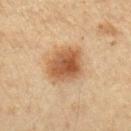– workup — imaged on a skin check; not biopsied
– automated lesion analysis — a lesion color around L≈49 a*≈19 b*≈33 in CIELAB, about 12 CIELAB-L* units darker than the surrounding skin, and a normalized border contrast of about 9; a border-irregularity rating of about 2/10, a within-lesion color-variation index near 5/10, and peripheral color asymmetry of about 1.5
– tile lighting — cross-polarized illumination
– image — ~15 mm tile from a whole-body skin photo
– diameter — ~4.5 mm (longest diameter)
– body site — the left forearm
– patient — female, about 45 years old